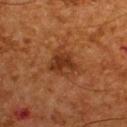biopsy_status: not biopsied; imaged during a skin examination
lesion_size:
  long_diameter_mm_approx: 4.0
automated_metrics:
  cielab_L: 29
  cielab_a: 21
  cielab_b: 29
  border_irregularity_0_10: 3.5
  color_variation_0_10: 4.0
  peripheral_color_asymmetry: 1.5
site: upper back
image:
  source: total-body photography crop
  field_of_view_mm: 15
lighting: cross-polarized
patient:
  sex: male
  age_approx: 65